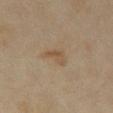Assessment: No biopsy was performed on this lesion — it was imaged during a full skin examination and was not determined to be concerning.